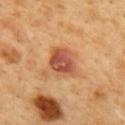Impression: Captured during whole-body skin photography for melanoma surveillance; the lesion was not biopsied. Acquisition and patient details: Imaged with cross-polarized lighting. Automated tile analysis of the lesion measured an area of roughly 9.5 mm², an eccentricity of roughly 0.7, and a shape-asymmetry score of about 0.2 (0 = symmetric). It also reported a nevus-likeness score of about 100/100 and a detector confidence of about 100 out of 100 that the crop contains a lesion. Longest diameter approximately 4 mm. A male patient, aged around 50. Cropped from a total-body skin-imaging series; the visible field is about 15 mm. Located on the mid back.